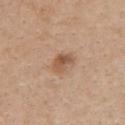Impression: Recorded during total-body skin imaging; not selected for excision or biopsy. Acquisition and patient details: The lesion is on the front of the torso. Cropped from a whole-body photographic skin survey; the tile spans about 15 mm. Automated tile analysis of the lesion measured a lesion area of about 4.5 mm² and two-axis asymmetry of about 0.3. The analysis additionally found a mean CIELAB color near L≈54 a*≈20 b*≈32 and a lesion-to-skin contrast of about 7.5 (normalized; higher = more distinct). The subject is a female roughly 30 years of age. Longest diameter approximately 3 mm.